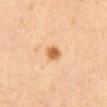The lesion was photographed on a routine skin check and not biopsied; there is no pathology result. The total-body-photography lesion software estimated a lesion area of about 3.5 mm², an eccentricity of roughly 0.7, and two-axis asymmetry of about 0.25. It also reported a color-variation rating of about 2.5/10 and peripheral color asymmetry of about 0.5. From the abdomen. Approximately 2.5 mm at its widest. Imaged with cross-polarized lighting. A close-up tile cropped from a whole-body skin photograph, about 15 mm across. A female patient approximately 55 years of age.